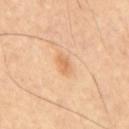Impression:
The lesion was photographed on a routine skin check and not biopsied; there is no pathology result.
Image and clinical context:
The lesion is on the mid back. A male subject aged 58 to 62. A region of skin cropped from a whole-body photographic capture, roughly 15 mm wide.